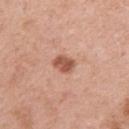Acquisition and patient details: A female patient aged 38–42. Located on the right upper arm. A roughly 15 mm field-of-view crop from a total-body skin photograph. Approximately 2.5 mm at its widest. Captured under white-light illumination.